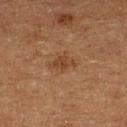biopsy status: imaged on a skin check; not biopsied | lesion size: about 2.5 mm | automated lesion analysis: a lesion area of about 4 mm², an outline eccentricity of about 0.65 (0 = round, 1 = elongated), and a shape-asymmetry score of about 0.35 (0 = symmetric); a border-irregularity rating of about 3.5/10, internal color variation of about 1 on a 0–10 scale, and radial color variation of about 0.5; a nevus-likeness score of about 15/100 and lesion-presence confidence of about 100/100 | subject: male, approximately 75 years of age | image: total-body-photography crop, ~15 mm field of view | location: the left lower leg | illumination: cross-polarized.